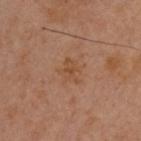The lesion is located on the upper back. A roughly 15 mm field-of-view crop from a total-body skin photograph. The tile uses cross-polarized illumination. A male patient, in their mid- to late 40s. The lesion-visualizer software estimated an average lesion color of about L≈47 a*≈21 b*≈32 (CIELAB), a lesion–skin lightness drop of about 6, and a normalized lesion–skin contrast near 5.5. It also reported a nevus-likeness score of about 0/100 and lesion-presence confidence of about 100/100. About 3 mm across.